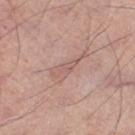Recorded during total-body skin imaging; not selected for excision or biopsy.
Imaged with white-light lighting.
This image is a 15 mm lesion crop taken from a total-body photograph.
Approximately 5 mm at its widest.
A male subject, aged 68–72.
An algorithmic analysis of the crop reported a lesion area of about 6.5 mm², an eccentricity of roughly 0.95, and a symmetry-axis asymmetry near 0.5. The software also gave an average lesion color of about L≈59 a*≈19 b*≈24 (CIELAB) and about 7 CIELAB-L* units darker than the surrounding skin. The analysis additionally found a color-variation rating of about 2.5/10 and radial color variation of about 0.5.
The lesion is on the left thigh.